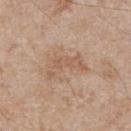This lesion was catalogued during total-body skin photography and was not selected for biopsy.
A male patient, in their mid- to late 60s.
From the chest.
A 15 mm close-up tile from a total-body photography series done for melanoma screening.